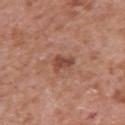Clinical impression: No biopsy was performed on this lesion — it was imaged during a full skin examination and was not determined to be concerning. Acquisition and patient details: A male subject, approximately 65 years of age. A 15 mm close-up extracted from a 3D total-body photography capture. The lesion is on the left upper arm.